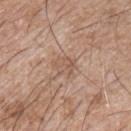Case summary:
- follow-up · total-body-photography surveillance lesion; no biopsy
- site · the front of the torso
- patient · male, roughly 65 years of age
- image-analysis metrics · border irregularity of about 3.5 on a 0–10 scale, a within-lesion color-variation index near 3/10, and peripheral color asymmetry of about 1
- tile lighting · white-light
- size · about 3 mm
- imaging modality · ~15 mm tile from a whole-body skin photo